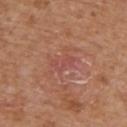Notes:
– workup — catalogued during a skin exam; not biopsied
– image — 15 mm crop, total-body photography
– patient — male, approximately 65 years of age
– location — the upper back
– illumination — white-light illumination